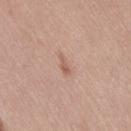Impression:
Part of a total-body skin-imaging series; this lesion was reviewed on a skin check and was not flagged for biopsy.
Acquisition and patient details:
The lesion-visualizer software estimated a lesion area of about 2.5 mm², a shape eccentricity near 0.9, and two-axis asymmetry of about 0.4. It also reported an average lesion color of about L≈59 a*≈20 b*≈29 (CIELAB) and a normalized lesion–skin contrast near 5.5. It also reported lesion-presence confidence of about 100/100. A female subject aged around 40. The lesion is on the right thigh. This image is a 15 mm lesion crop taken from a total-body photograph.